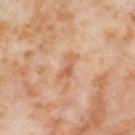Assessment:
The lesion was tiled from a total-body skin photograph and was not biopsied.
Image and clinical context:
A close-up tile cropped from a whole-body skin photograph, about 15 mm across. About 3.5 mm across. Imaged with cross-polarized lighting. The lesion is on the left thigh. A female subject, aged around 55.